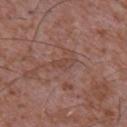Notes:
- biopsy status — imaged on a skin check; not biopsied